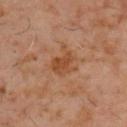- follow-up · imaged on a skin check; not biopsied
- subject · male, roughly 60 years of age
- lighting · cross-polarized illumination
- location · the back
- acquisition · 15 mm crop, total-body photography
- lesion diameter · ~4 mm (longest diameter)
- automated metrics · an area of roughly 7.5 mm², an eccentricity of roughly 0.7, and two-axis asymmetry of about 0.35; a color-variation rating of about 2.5/10 and peripheral color asymmetry of about 0.5; an automated nevus-likeness rating near 5 out of 100 and lesion-presence confidence of about 100/100